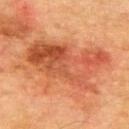notes: total-body-photography surveillance lesion; no biopsy | subject: male, aged 73–77 | anatomic site: the upper back | automated metrics: a lesion area of about 43 mm², a shape eccentricity near 0.85, and two-axis asymmetry of about 0.4; a lesion color around L≈45 a*≈27 b*≈33 in CIELAB and roughly 10 lightness units darker than nearby skin | imaging modality: ~15 mm tile from a whole-body skin photo.